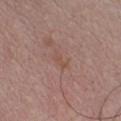Clinical summary:
From the chest. A male subject in their mid-40s. About 2.5 mm across. This image is a 15 mm lesion crop taken from a total-body photograph. Captured under white-light illumination. An algorithmic analysis of the crop reported an average lesion color of about L≈50 a*≈20 b*≈26 (CIELAB), about 5 CIELAB-L* units darker than the surrounding skin, and a lesion-to-skin contrast of about 5 (normalized; higher = more distinct). The analysis additionally found a classifier nevus-likeness of about 0/100.